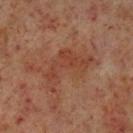A male patient, aged 58 to 62. A lesion tile, about 15 mm wide, cut from a 3D total-body photograph. On the left lower leg.